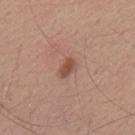Impression: Recorded during total-body skin imaging; not selected for excision or biopsy. Context: On the upper back. The subject is a male in their mid-50s. A 15 mm crop from a total-body photograph taken for skin-cancer surveillance. The lesion-visualizer software estimated a shape eccentricity near 0.8 and two-axis asymmetry of about 0.4. The analysis additionally found a border-irregularity rating of about 3/10 and a color-variation rating of about 2/10. The software also gave an automated nevus-likeness rating near 90 out of 100. This is a white-light tile. About 2.5 mm across.